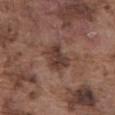workup = total-body-photography surveillance lesion; no biopsy
subject = male, aged around 75
lighting = white-light
site = the front of the torso
size = ~3.5 mm (longest diameter)
automated lesion analysis = an area of roughly 8 mm² and an eccentricity of roughly 0.7; a within-lesion color-variation index near 3.5/10 and peripheral color asymmetry of about 1; an automated nevus-likeness rating near 0 out of 100
image source = total-body-photography crop, ~15 mm field of view